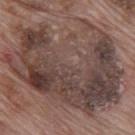<case>
<biopsy_status>not biopsied; imaged during a skin examination</biopsy_status>
<site>mid back</site>
<image>
  <source>total-body photography crop</source>
  <field_of_view_mm>15</field_of_view_mm>
</image>
<patient>
  <sex>male</sex>
  <age_approx>70</age_approx>
</patient>
<automated_metrics>
  <area_mm2_approx>90.0</area_mm2_approx>
  <nevus_likeness_0_100>0</nevus_likeness_0_100>
  <lesion_detection_confidence_0_100>50</lesion_detection_confidence_0_100>
</automated_metrics>
</case>